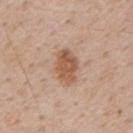Q: Was this lesion biopsied?
A: total-body-photography surveillance lesion; no biopsy